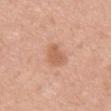biopsy status: total-body-photography surveillance lesion; no biopsy | diameter: ≈3.5 mm | automated metrics: a lesion area of about 5 mm², an outline eccentricity of about 0.85 (0 = round, 1 = elongated), and a symmetry-axis asymmetry near 0.3; a color-variation rating of about 2/10; a nevus-likeness score of about 10/100 | imaging modality: 15 mm crop, total-body photography | site: the upper back | patient: female, about 35 years old | tile lighting: white-light.